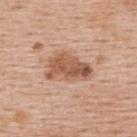Cropped from a total-body skin-imaging series; the visible field is about 15 mm.
The subject is a female roughly 65 years of age.
Captured under white-light illumination.
From the back.